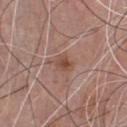Q: Lesion size?
A: ≈2.5 mm
Q: How was the tile lit?
A: white-light illumination
Q: Patient demographics?
A: male, approximately 75 years of age
Q: What kind of image is this?
A: 15 mm crop, total-body photography
Q: Automated lesion metrics?
A: an area of roughly 3.5 mm², an outline eccentricity of about 0.65 (0 = round, 1 = elongated), and a shape-asymmetry score of about 0.15 (0 = symmetric); a lesion color around L≈48 a*≈21 b*≈27 in CIELAB and a lesion-to-skin contrast of about 7 (normalized; higher = more distinct)
Q: Where on the body is the lesion?
A: the front of the torso